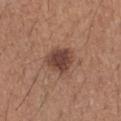Imaged during a routine full-body skin examination; the lesion was not biopsied and no histopathology is available.
Captured under white-light illumination.
A female patient, aged approximately 25.
On the arm.
Longest diameter approximately 3.5 mm.
A close-up tile cropped from a whole-body skin photograph, about 15 mm across.
An algorithmic analysis of the crop reported a lesion area of about 9 mm², an outline eccentricity of about 0.5 (0 = round, 1 = elongated), and two-axis asymmetry of about 0.2. The software also gave a color-variation rating of about 3/10 and a peripheral color-asymmetry measure near 1.